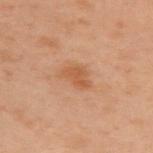notes = no biopsy performed (imaged during a skin exam).